From the left thigh, a male subject aged 58–62, a 15 mm close-up tile from a total-body photography series done for melanoma screening, the lesion's longest dimension is about 9.5 mm:
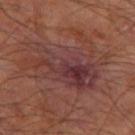Pathology:
The biopsy diagnosis was an atypical melanocytic neoplasm, classified as an indeterminate (borderline) lesion.